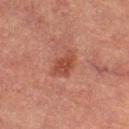Captured during whole-body skin photography for melanoma surveillance; the lesion was not biopsied.